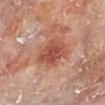workup: total-body-photography surveillance lesion; no biopsy
location: the left lower leg
imaging modality: 15 mm crop, total-body photography
lighting: cross-polarized illumination
diameter: ≈4 mm
patient: male, in their 60s